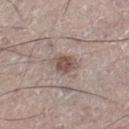Q: Was a biopsy performed?
A: catalogued during a skin exam; not biopsied
Q: What did automated image analysis measure?
A: a footprint of about 5.5 mm², an eccentricity of roughly 0.7, and a shape-asymmetry score of about 0.2 (0 = symmetric); a lesion color around L≈51 a*≈16 b*≈22 in CIELAB, a lesion–skin lightness drop of about 11, and a normalized border contrast of about 8; border irregularity of about 2 on a 0–10 scale and a color-variation rating of about 2.5/10
Q: What are the patient's age and sex?
A: male, aged approximately 70
Q: Where on the body is the lesion?
A: the left leg
Q: What is the lesion's diameter?
A: ~3 mm (longest diameter)
Q: What is the imaging modality?
A: ~15 mm tile from a whole-body skin photo